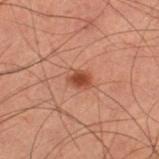lesion_size:
  long_diameter_mm_approx: 2.5
site: left thigh
image:
  source: total-body photography crop
  field_of_view_mm: 15
patient:
  sex: male
  age_approx: 60